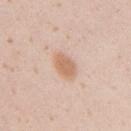| field | value |
|---|---|
| notes | imaged on a skin check; not biopsied |
| subject | female, roughly 20 years of age |
| acquisition | ~15 mm tile from a whole-body skin photo |
| size | ≈3 mm |
| site | the right upper arm |
| TBP lesion metrics | roughly 10 lightness units darker than nearby skin and a normalized border contrast of about 7.5; border irregularity of about 2 on a 0–10 scale, internal color variation of about 1.5 on a 0–10 scale, and peripheral color asymmetry of about 0.5 |